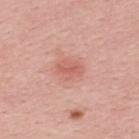{"biopsy_status": "not biopsied; imaged during a skin examination", "image": {"source": "total-body photography crop", "field_of_view_mm": 15}, "lighting": "white-light", "lesion_size": {"long_diameter_mm_approx": 3.0}, "patient": {"sex": "male", "age_approx": 30}, "site": "upper back", "automated_metrics": {"area_mm2_approx": 5.5, "eccentricity": 0.75, "shape_asymmetry": 0.2, "border_irregularity_0_10": 2.0, "color_variation_0_10": 2.5, "nevus_likeness_0_100": 40, "lesion_detection_confidence_0_100": 100}}